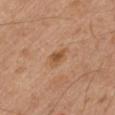The lesion was photographed on a routine skin check and not biopsied; there is no pathology result.
The total-body-photography lesion software estimated lesion-presence confidence of about 100/100.
This is a cross-polarized tile.
The patient is a male about 45 years old.
The lesion is located on the left upper arm.
The recorded lesion diameter is about 3 mm.
A close-up tile cropped from a whole-body skin photograph, about 15 mm across.